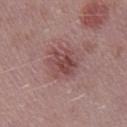Clinical impression:
No biopsy was performed on this lesion — it was imaged during a full skin examination and was not determined to be concerning.
Background:
From the right lower leg. A male subject, aged approximately 40. The total-body-photography lesion software estimated an outline eccentricity of about 0.45 (0 = round, 1 = elongated) and a symmetry-axis asymmetry near 0.2. And it measured a lesion–skin lightness drop of about 9. Longest diameter approximately 4 mm. Imaged with white-light lighting. A region of skin cropped from a whole-body photographic capture, roughly 15 mm wide.